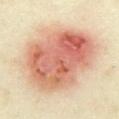Captured during whole-body skin photography for melanoma surveillance; the lesion was not biopsied. Cropped from a whole-body photographic skin survey; the tile spans about 15 mm. About 10 mm across. An algorithmic analysis of the crop reported a lesion area of about 60 mm² and a shape eccentricity near 0.65. A female patient, aged around 35. From the abdomen.